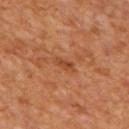workup: imaged on a skin check; not biopsied | image source: ~15 mm tile from a whole-body skin photo | diameter: ~3 mm (longest diameter) | anatomic site: the mid back | TBP lesion metrics: a mean CIELAB color near L≈44 a*≈25 b*≈36 and a lesion-to-skin contrast of about 5.5 (normalized; higher = more distinct); a border-irregularity rating of about 2.5/10, internal color variation of about 2 on a 0–10 scale, and peripheral color asymmetry of about 0.5; a nevus-likeness score of about 0/100 and lesion-presence confidence of about 100/100 | lighting: cross-polarized | patient: male, aged 63–67.